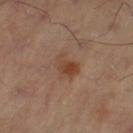The lesion was tiled from a total-body skin photograph and was not biopsied. The tile uses cross-polarized illumination. A 15 mm close-up tile from a total-body photography series done for melanoma screening. From the left thigh. The total-body-photography lesion software estimated a border-irregularity index near 3/10, a color-variation rating of about 5/10, and a peripheral color-asymmetry measure near 1.5. Measured at roughly 3.5 mm in maximum diameter.